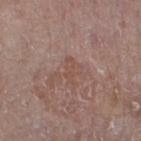Recorded during total-body skin imaging; not selected for excision or biopsy. The lesion-visualizer software estimated a footprint of about 3 mm² and an eccentricity of roughly 0.95. The analysis additionally found a border-irregularity rating of about 5.5/10, a within-lesion color-variation index near 0/10, and radial color variation of about 0. Captured under white-light illumination. A close-up tile cropped from a whole-body skin photograph, about 15 mm across. Longest diameter approximately 3 mm. A female patient, aged around 85 or older. The lesion is located on the left lower leg.